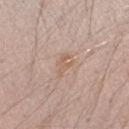This lesion was catalogued during total-body skin photography and was not selected for biopsy. A male patient, approximately 40 years of age. About 3 mm across. This is a white-light tile. Located on the right forearm. The total-body-photography lesion software estimated an average lesion color of about L≈60 a*≈17 b*≈28 (CIELAB), roughly 7 lightness units darker than nearby skin, and a normalized border contrast of about 5.5. The analysis additionally found a border-irregularity rating of about 5.5/10 and a color-variation rating of about 0/10. The software also gave an automated nevus-likeness rating near 0 out of 100 and a lesion-detection confidence of about 100/100. A roughly 15 mm field-of-view crop from a total-body skin photograph.